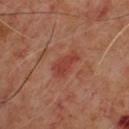workup = imaged on a skin check; not biopsied
patient = male, in their mid-60s
illumination = cross-polarized
acquisition = 15 mm crop, total-body photography
diameter = ~3.5 mm (longest diameter)